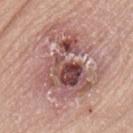Notes:
- notes · no biopsy performed (imaged during a skin exam)